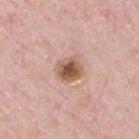{
  "lesion_size": {
    "long_diameter_mm_approx": 3.5
  },
  "image": {
    "source": "total-body photography crop",
    "field_of_view_mm": 15
  },
  "lighting": "white-light",
  "site": "front of the torso",
  "patient": {
    "sex": "male",
    "age_approx": 60
  }
}